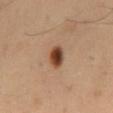notes: no biopsy performed (imaged during a skin exam) | location: the back | image source: 15 mm crop, total-body photography | TBP lesion metrics: an area of roughly 5.5 mm², an outline eccentricity of about 0.8 (0 = round, 1 = elongated), and a shape-asymmetry score of about 0.1 (0 = symmetric); an automated nevus-likeness rating near 100 out of 100 and a detector confidence of about 100 out of 100 that the crop contains a lesion | diameter: about 3.5 mm | subject: male, about 40 years old.